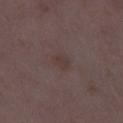Clinical impression:
No biopsy was performed on this lesion — it was imaged during a full skin examination and was not determined to be concerning.
Acquisition and patient details:
Imaged with white-light lighting. From the right thigh. Cropped from a whole-body photographic skin survey; the tile spans about 15 mm. A female patient about 35 years old. About 2.5 mm across.